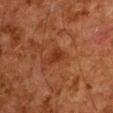{"biopsy_status": "not biopsied; imaged during a skin examination", "image": {"source": "total-body photography crop", "field_of_view_mm": 15}, "patient": {"sex": "male", "age_approx": 60}, "lesion_size": {"long_diameter_mm_approx": 2.5}, "site": "chest", "lighting": "cross-polarized", "automated_metrics": {"eccentricity": 0.8, "shape_asymmetry": 0.35, "cielab_L": 26, "cielab_a": 23, "cielab_b": 29, "vs_skin_contrast_norm": 7.0}}